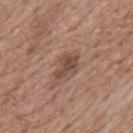Findings:
- notes — imaged on a skin check; not biopsied
- image — 15 mm crop, total-body photography
- location — the mid back
- patient — male, roughly 70 years of age